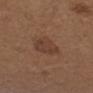No biopsy was performed on this lesion — it was imaged during a full skin examination and was not determined to be concerning.
A female patient in their 40s.
A 15 mm crop from a total-body photograph taken for skin-cancer surveillance.
On the left forearm.